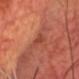biopsy status — no biopsy performed (imaged during a skin exam) | illumination — cross-polarized illumination | anatomic site — the head or neck | patient — male, aged 68–72 | automated lesion analysis — a nevus-likeness score of about 0/100 | diameter — ≈3 mm | image source — ~15 mm crop, total-body skin-cancer survey.